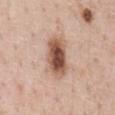The lesion was photographed on a routine skin check and not biopsied; there is no pathology result.
A female subject, approximately 45 years of age.
From the chest.
A 15 mm close-up tile from a total-body photography series done for melanoma screening.